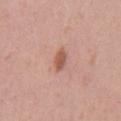workup: imaged on a skin check; not biopsied | tile lighting: white-light illumination | image: total-body-photography crop, ~15 mm field of view | location: the front of the torso | size: ≈2.5 mm | patient: male, roughly 55 years of age.